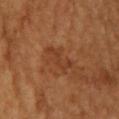biopsy status = imaged on a skin check; not biopsied | body site = the back | subject = male, aged 63–67 | acquisition = ~15 mm crop, total-body skin-cancer survey.